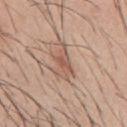{
  "biopsy_status": "not biopsied; imaged during a skin examination",
  "patient": {
    "sex": "male",
    "age_approx": 45
  },
  "lighting": "white-light",
  "site": "chest",
  "image": {
    "source": "total-body photography crop",
    "field_of_view_mm": 15
  },
  "automated_metrics": {
    "area_mm2_approx": 8.0,
    "eccentricity": 0.85,
    "shape_asymmetry": 0.4,
    "cielab_L": 57,
    "cielab_a": 19,
    "cielab_b": 28,
    "vs_skin_darker_L": 10.0,
    "nevus_likeness_0_100": 0,
    "lesion_detection_confidence_0_100": 100
  }
}